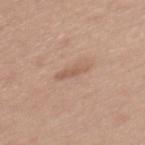Recorded during total-body skin imaging; not selected for excision or biopsy. Automated image analysis of the tile measured a lesion–skin lightness drop of about 8 and a normalized lesion–skin contrast near 5.5. And it measured a border-irregularity rating of about 3.5/10 and a peripheral color-asymmetry measure near 0. The software also gave a classifier nevus-likeness of about 0/100 and lesion-presence confidence of about 100/100. The lesion's longest dimension is about 3.5 mm. Imaged with white-light lighting. A female patient, approximately 35 years of age. From the mid back. This image is a 15 mm lesion crop taken from a total-body photograph.